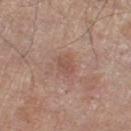Q: Was this lesion biopsied?
A: total-body-photography surveillance lesion; no biopsy
Q: What did automated image analysis measure?
A: a lesion area of about 4.5 mm², a shape eccentricity near 0.65, and two-axis asymmetry of about 0.2; a mean CIELAB color near L≈52 a*≈20 b*≈26, a lesion–skin lightness drop of about 7, and a lesion-to-skin contrast of about 5 (normalized; higher = more distinct); a border-irregularity rating of about 2/10, a within-lesion color-variation index near 1.5/10, and radial color variation of about 0.5; a nevus-likeness score of about 0/100 and lesion-presence confidence of about 100/100
Q: What is the lesion's diameter?
A: about 2.5 mm
Q: How was this image acquired?
A: ~15 mm tile from a whole-body skin photo
Q: Illumination type?
A: white-light illumination
Q: What are the patient's age and sex?
A: male, approximately 80 years of age
Q: What is the anatomic site?
A: the left thigh